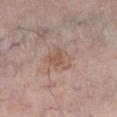Part of a total-body skin-imaging series; this lesion was reviewed on a skin check and was not flagged for biopsy.
Captured under white-light illumination.
The lesion is located on the right lower leg.
The subject is a male aged 58 to 62.
A region of skin cropped from a whole-body photographic capture, roughly 15 mm wide.
Measured at roughly 3.5 mm in maximum diameter.
The total-body-photography lesion software estimated a lesion area of about 7.5 mm² and a symmetry-axis asymmetry near 0.35. And it measured a lesion–skin lightness drop of about 7 and a normalized border contrast of about 5.5. And it measured border irregularity of about 3 on a 0–10 scale. And it measured a classifier nevus-likeness of about 0/100 and a detector confidence of about 100 out of 100 that the crop contains a lesion.